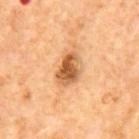  image:
    source: total-body photography crop
    field_of_view_mm: 15
  lighting: cross-polarized
  lesion_size:
    long_diameter_mm_approx: 4.5
  site: mid back
  automated_metrics:
    cielab_L: 57
    cielab_a: 23
    cielab_b: 40
    nevus_likeness_0_100: 90
  patient:
    sex: male
    age_approx: 65